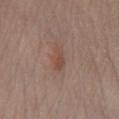workup = total-body-photography surveillance lesion; no biopsy
diameter = ~3 mm (longest diameter)
image source = total-body-photography crop, ~15 mm field of view
patient = male, approximately 60 years of age
illumination = white-light
site = the leg